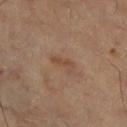{
  "biopsy_status": "not biopsied; imaged during a skin examination",
  "site": "left leg",
  "image": {
    "source": "total-body photography crop",
    "field_of_view_mm": 15
  },
  "patient": {
    "sex": "female",
    "age_approx": 60
  }
}